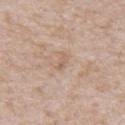{"patient": {"sex": "male", "age_approx": 65}, "image": {"source": "total-body photography crop", "field_of_view_mm": 15}, "site": "abdomen", "lesion_size": {"long_diameter_mm_approx": 2.5}}